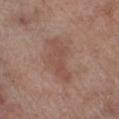This lesion was catalogued during total-body skin photography and was not selected for biopsy. Cropped from a total-body skin-imaging series; the visible field is about 15 mm. Approximately 6 mm at its widest. The patient is a male in their 70s. From the left lower leg. The tile uses white-light illumination.